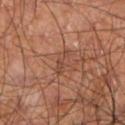The lesion was tiled from a total-body skin photograph and was not biopsied. A roughly 15 mm field-of-view crop from a total-body skin photograph. A male subject approximately 60 years of age. The lesion's longest dimension is about 3.5 mm. Located on the left lower leg. This is a cross-polarized tile. The lesion-visualizer software estimated a lesion area of about 3 mm² and two-axis asymmetry of about 0.45. The software also gave a mean CIELAB color near L≈44 a*≈22 b*≈29 and a normalized border contrast of about 5.5. The analysis additionally found a border-irregularity index near 5.5/10 and radial color variation of about 0. And it measured a classifier nevus-likeness of about 0/100 and lesion-presence confidence of about 60/100.